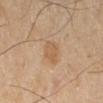  biopsy_status: not biopsied; imaged during a skin examination
  site: right lower leg
  automated_metrics:
    area_mm2_approx: 5.0
    eccentricity: 0.7
    shape_asymmetry: 0.2
    nevus_likeness_0_100: 55
  image:
    source: total-body photography crop
    field_of_view_mm: 15
  patient:
    sex: female
    age_approx: 70
  lesion_size:
    long_diameter_mm_approx: 2.5